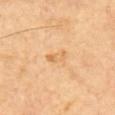image: ~15 mm tile from a whole-body skin photo; body site: the upper back; subject: male, aged approximately 60.